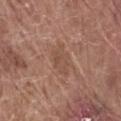workup = no biopsy performed (imaged during a skin exam)
diameter = ~3.5 mm (longest diameter)
patient = male, roughly 80 years of age
location = the front of the torso
imaging modality = 15 mm crop, total-body photography
tile lighting = white-light illumination